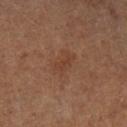  image:
    source: total-body photography crop
    field_of_view_mm: 15
  site: left lower leg
  lighting: cross-polarized
  patient:
    sex: male
    age_approx: 60
  lesion_size:
    long_diameter_mm_approx: 3.5